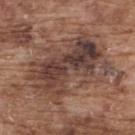biopsy status: no biopsy performed (imaged during a skin exam) | imaging modality: total-body-photography crop, ~15 mm field of view | location: the back | patient: male, in their mid- to late 70s | tile lighting: white-light illumination | automated metrics: an area of roughly 41 mm² and an outline eccentricity of about 0.8 (0 = round, 1 = elongated); a lesion color around L≈42 a*≈18 b*≈23 in CIELAB and a lesion–skin lightness drop of about 10.